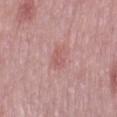Recorded during total-body skin imaging; not selected for excision or biopsy. The subject is a female aged approximately 70. Located on the right thigh. Cropped from a whole-body photographic skin survey; the tile spans about 15 mm. An algorithmic analysis of the crop reported a color-variation rating of about 2/10 and peripheral color asymmetry of about 0.5. And it measured a classifier nevus-likeness of about 0/100 and lesion-presence confidence of about 100/100.